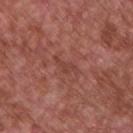The lesion was tiled from a total-body skin photograph and was not biopsied.
From the chest.
A male subject, aged around 65.
A 15 mm close-up tile from a total-body photography series done for melanoma screening.
Longest diameter approximately 3.5 mm.